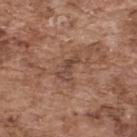This lesion was catalogued during total-body skin photography and was not selected for biopsy. The tile uses white-light illumination. A close-up tile cropped from a whole-body skin photograph, about 15 mm across. Automated image analysis of the tile measured a lesion area of about 5 mm², a shape eccentricity near 0.85, and a symmetry-axis asymmetry near 0.45. The analysis additionally found a border-irregularity index near 5.5/10 and peripheral color asymmetry of about 0.5. The software also gave a lesion-detection confidence of about 90/100. Located on the upper back. The subject is a male aged 73 to 77. Approximately 3.5 mm at its widest.